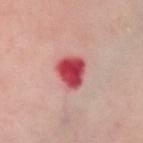Clinical impression: Imaged during a routine full-body skin examination; the lesion was not biopsied and no histopathology is available. Background: A female patient, aged approximately 55. Measured at roughly 4 mm in maximum diameter. Cropped from a total-body skin-imaging series; the visible field is about 15 mm. Located on the chest. Captured under cross-polarized illumination.